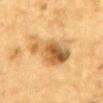Assessment:
No biopsy was performed on this lesion — it was imaged during a full skin examination and was not determined to be concerning.
Image and clinical context:
Captured under cross-polarized illumination. A lesion tile, about 15 mm wide, cut from a 3D total-body photograph. The subject is a male in their mid- to late 80s or older. Located on the upper back.